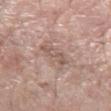biopsy_status: not biopsied; imaged during a skin examination
site: left forearm
image:
  source: total-body photography crop
  field_of_view_mm: 15
patient:
  sex: male
  age_approx: 60
lesion_size:
  long_diameter_mm_approx: 3.5
automated_metrics:
  border_irregularity_0_10: 5.0
  color_variation_0_10: 1.5
  peripheral_color_asymmetry: 0.5
  nevus_likeness_0_100: 0
  lesion_detection_confidence_0_100: 95